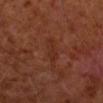workup — no biopsy performed (imaged during a skin exam)
anatomic site — the right forearm
lesion size — ≈4 mm
patient — male, aged 58–62
image — 15 mm crop, total-body photography
automated lesion analysis — a mean CIELAB color near L≈27 a*≈23 b*≈27, roughly 4 lightness units darker than nearby skin, and a normalized lesion–skin contrast near 4.5; a border-irregularity index near 3/10, internal color variation of about 2 on a 0–10 scale, and peripheral color asymmetry of about 0.5